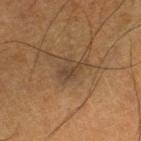The lesion was tiled from a total-body skin photograph and was not biopsied.
Cropped from a whole-body photographic skin survey; the tile spans about 15 mm.
Measured at roughly 2.5 mm in maximum diameter.
On the right lower leg.
Captured under cross-polarized illumination.
A male patient aged 53 to 57.